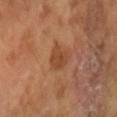Q: Was a biopsy performed?
A: total-body-photography surveillance lesion; no biopsy
Q: Lesion size?
A: ≈3 mm
Q: What kind of image is this?
A: ~15 mm tile from a whole-body skin photo
Q: What did automated image analysis measure?
A: an area of roughly 5.5 mm², an eccentricity of roughly 0.65, and a symmetry-axis asymmetry near 0.25; an average lesion color of about L≈44 a*≈23 b*≈34 (CIELAB), about 8 CIELAB-L* units darker than the surrounding skin, and a normalized lesion–skin contrast near 6.5
Q: Patient demographics?
A: male, roughly 65 years of age
Q: How was the tile lit?
A: cross-polarized